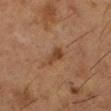Acquisition and patient details: Approximately 2.5 mm at its widest. Captured under cross-polarized illumination. A 15 mm crop from a total-body photograph taken for skin-cancer surveillance. A female subject, aged approximately 50. On the left lower leg.